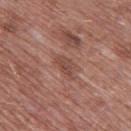{
  "biopsy_status": "not biopsied; imaged during a skin examination",
  "image": {
    "source": "total-body photography crop",
    "field_of_view_mm": 15
  },
  "lighting": "white-light",
  "automated_metrics": {
    "nevus_likeness_0_100": 0
  },
  "lesion_size": {
    "long_diameter_mm_approx": 2.5
  },
  "site": "right thigh",
  "patient": {
    "sex": "male",
    "age_approx": 60
  }
}